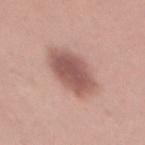Imaged during a routine full-body skin examination; the lesion was not biopsied and no histopathology is available.
A 15 mm close-up tile from a total-body photography series done for melanoma screening.
The subject is a female in their mid- to late 20s.
From the mid back.
The tile uses white-light illumination.
Longest diameter approximately 6 mm.